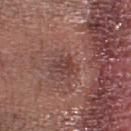Recorded during total-body skin imaging; not selected for excision or biopsy.
A female subject, aged 53–57.
Located on the head or neck.
The tile uses white-light illumination.
A region of skin cropped from a whole-body photographic capture, roughly 15 mm wide.